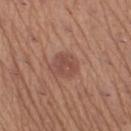biopsy status=imaged on a skin check; not biopsied
automated metrics=about 8 CIELAB-L* units darker than the surrounding skin and a normalized lesion–skin contrast near 6; border irregularity of about 2 on a 0–10 scale, a within-lesion color-variation index near 2/10, and peripheral color asymmetry of about 1
site=the left lower leg
imaging modality=15 mm crop, total-body photography
patient=female, roughly 65 years of age